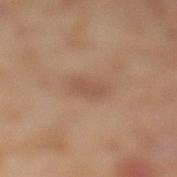Part of a total-body skin-imaging series; this lesion was reviewed on a skin check and was not flagged for biopsy. Longest diameter approximately 3 mm. A female patient, aged 53 to 57. From the left lower leg. Cropped from a total-body skin-imaging series; the visible field is about 15 mm.